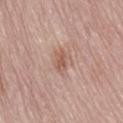{"biopsy_status": "not biopsied; imaged during a skin examination", "patient": {"sex": "female", "age_approx": 65}, "lighting": "white-light", "automated_metrics": {"area_mm2_approx": 3.5, "eccentricity": 0.85, "shape_asymmetry": 0.25, "cielab_L": 56, "cielab_a": 21, "cielab_b": 27, "vs_skin_contrast_norm": 6.5, "nevus_likeness_0_100": 0}, "site": "lower back", "lesion_size": {"long_diameter_mm_approx": 3.0}, "image": {"source": "total-body photography crop", "field_of_view_mm": 15}}